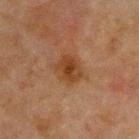Findings:
* biopsy status — imaged on a skin check; not biopsied
* acquisition — ~15 mm tile from a whole-body skin photo
* patient — male, aged 63 to 67
* TBP lesion metrics — a lesion color around L≈33 a*≈19 b*≈30 in CIELAB, a lesion–skin lightness drop of about 8, and a lesion-to-skin contrast of about 8.5 (normalized; higher = more distinct)
* illumination — cross-polarized
* anatomic site — the chest